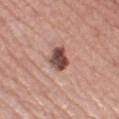No biopsy was performed on this lesion — it was imaged during a full skin examination and was not determined to be concerning.